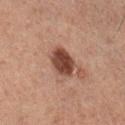The lesion was tiled from a total-body skin photograph and was not biopsied. Imaged with cross-polarized lighting. This image is a 15 mm lesion crop taken from a total-body photograph. The patient is a male roughly 60 years of age. An algorithmic analysis of the crop reported an eccentricity of roughly 0.5 and two-axis asymmetry of about 0.1. The software also gave a nevus-likeness score of about 90/100 and lesion-presence confidence of about 100/100. The lesion is located on the right lower leg.